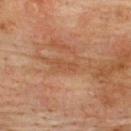Notes:
- imaging modality: ~15 mm tile from a whole-body skin photo
- diameter: ≈2.5 mm
- automated lesion analysis: a lesion–skin lightness drop of about 5 and a normalized border contrast of about 4.5; border irregularity of about 3.5 on a 0–10 scale, a within-lesion color-variation index near 0/10, and a peripheral color-asymmetry measure near 0; an automated nevus-likeness rating near 0 out of 100 and lesion-presence confidence of about 90/100
- lighting: cross-polarized
- location: the upper back
- subject: male, about 75 years old